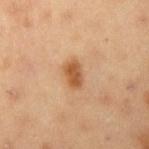Q: Was this lesion biopsied?
A: no biopsy performed (imaged during a skin exam)
Q: How large is the lesion?
A: ~3 mm (longest diameter)
Q: How was the tile lit?
A: cross-polarized illumination
Q: What kind of image is this?
A: ~15 mm crop, total-body skin-cancer survey
Q: Patient demographics?
A: male, in their mid-50s
Q: What did automated image analysis measure?
A: a lesion-to-skin contrast of about 8.5 (normalized; higher = more distinct)
Q: What is the anatomic site?
A: the right upper arm